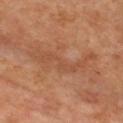notes: imaged on a skin check; not biopsied
lesion size: about 8 mm
image-analysis metrics: a mean CIELAB color near L≈48 a*≈23 b*≈33 and a lesion-to-skin contrast of about 5 (normalized; higher = more distinct)
illumination: cross-polarized
patient: female, in their 50s
body site: the chest
imaging modality: ~15 mm tile from a whole-body skin photo